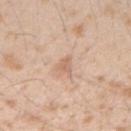Impression:
The lesion was tiled from a total-body skin photograph and was not biopsied.
Image and clinical context:
A 15 mm close-up tile from a total-body photography series done for melanoma screening. The lesion is located on the right forearm. A male subject, aged approximately 25. Imaged with white-light lighting.